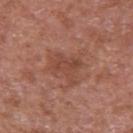follow-up: catalogued during a skin exam; not biopsied
imaging modality: total-body-photography crop, ~15 mm field of view
subject: male, in their mid- to late 60s
image-analysis metrics: a lesion color around L≈46 a*≈24 b*≈28 in CIELAB, about 7 CIELAB-L* units darker than the surrounding skin, and a normalized border contrast of about 5.5; a border-irregularity rating of about 5/10 and internal color variation of about 3 on a 0–10 scale
lesion size: ≈4 mm
lighting: white-light
location: the right upper arm